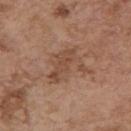No biopsy was performed on this lesion — it was imaged during a full skin examination and was not determined to be concerning.
A 15 mm close-up tile from a total-body photography series done for melanoma screening.
About 5 mm across.
The total-body-photography lesion software estimated a border-irregularity rating of about 8.5/10, a color-variation rating of about 3/10, and radial color variation of about 1. The analysis additionally found an automated nevus-likeness rating near 0 out of 100 and a lesion-detection confidence of about 100/100.
On the arm.
This is a white-light tile.
A female patient, aged 63–67.